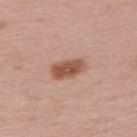| field | value |
|---|---|
| notes | no biopsy performed (imaged during a skin exam) |
| illumination | white-light |
| site | the upper back |
| acquisition | total-body-photography crop, ~15 mm field of view |
| subject | female, about 50 years old |